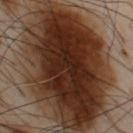Captured during whole-body skin photography for melanoma surveillance; the lesion was not biopsied. The lesion is on the chest. A male subject, aged 53 to 57. A 15 mm close-up extracted from a 3D total-body photography capture.